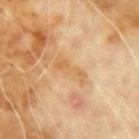Findings:
• workup: no biopsy performed (imaged during a skin exam)
• illumination: cross-polarized
• acquisition: ~15 mm tile from a whole-body skin photo
• diameter: ~4.5 mm (longest diameter)
• location: the left upper arm
• subject: male, aged 68 to 72